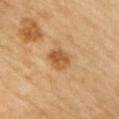{"biopsy_status": "not biopsied; imaged during a skin examination", "site": "chest", "automated_metrics": {"border_irregularity_0_10": 1.5, "color_variation_0_10": 4.5}, "patient": {"sex": "female", "age_approx": 60}, "image": {"source": "total-body photography crop", "field_of_view_mm": 15}}